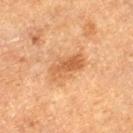Q: What kind of image is this?
A: ~15 mm crop, total-body skin-cancer survey
Q: What is the lesion's diameter?
A: ≈4 mm
Q: Where on the body is the lesion?
A: the leg
Q: Who is the patient?
A: male, roughly 75 years of age